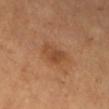No biopsy was performed on this lesion — it was imaged during a full skin examination and was not determined to be concerning. This image is a 15 mm lesion crop taken from a total-body photograph. Imaged with cross-polarized lighting. Measured at roughly 3.5 mm in maximum diameter. On the left lower leg. A female patient, about 30 years old.